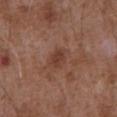{
  "biopsy_status": "not biopsied; imaged during a skin examination",
  "site": "abdomen",
  "patient": {
    "sex": "male",
    "age_approx": 75
  },
  "image": {
    "source": "total-body photography crop",
    "field_of_view_mm": 15
  }
}